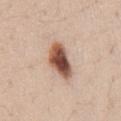workup=total-body-photography surveillance lesion; no biopsy | site=the lower back | tile lighting=white-light | subject=male, aged 33–37 | imaging modality=~15 mm crop, total-body skin-cancer survey | diameter=~5 mm (longest diameter) | automated lesion analysis=a lesion color around L≈52 a*≈22 b*≈29 in CIELAB and a normalized border contrast of about 13.5.